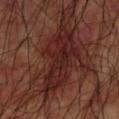Case summary:
- workup — imaged on a skin check; not biopsied
- illumination — cross-polarized illumination
- TBP lesion metrics — a lesion color around L≈23 a*≈23 b*≈21 in CIELAB, roughly 8 lightness units darker than nearby skin, and a normalized border contrast of about 9; a within-lesion color-variation index near 3.5/10 and peripheral color asymmetry of about 1; an automated nevus-likeness rating near 0 out of 100 and lesion-presence confidence of about 95/100
- imaging modality — 15 mm crop, total-body photography
- patient — male, aged 58 to 62
- body site — the left upper arm
- lesion size — ~9 mm (longest diameter)